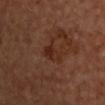Q: Was this lesion biopsied?
A: catalogued during a skin exam; not biopsied
Q: Lesion location?
A: the chest
Q: Illumination type?
A: cross-polarized illumination
Q: What is the lesion's diameter?
A: ≈3.5 mm
Q: How was this image acquired?
A: total-body-photography crop, ~15 mm field of view
Q: Patient demographics?
A: male, roughly 70 years of age